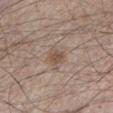Notes:
- follow-up · catalogued during a skin exam; not biopsied
- acquisition · 15 mm crop, total-body photography
- anatomic site · the left lower leg
- lighting · white-light
- size · ≈2.5 mm
- subject · male, approximately 45 years of age
- automated lesion analysis · a lesion area of about 4.5 mm², a shape eccentricity near 0.5, and two-axis asymmetry of about 0.2; an average lesion color of about L≈51 a*≈16 b*≈25 (CIELAB) and a lesion–skin lightness drop of about 8